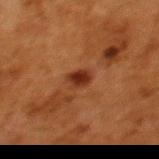Recorded during total-body skin imaging; not selected for excision or biopsy.